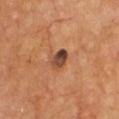Impression: This lesion was catalogued during total-body skin photography and was not selected for biopsy. Background: An algorithmic analysis of the crop reported a lesion area of about 4.5 mm², an eccentricity of roughly 0.65, and two-axis asymmetry of about 0.15. The software also gave a mean CIELAB color near L≈46 a*≈22 b*≈31, a lesion–skin lightness drop of about 15, and a normalized lesion–skin contrast near 11. The software also gave a border-irregularity rating of about 1.5/10, internal color variation of about 7 on a 0–10 scale, and radial color variation of about 2.5. And it measured an automated nevus-likeness rating near 0 out of 100. Approximately 2.5 mm at its widest. A 15 mm crop from a total-body photograph taken for skin-cancer surveillance. The lesion is located on the chest.